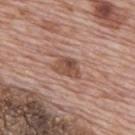Clinical impression: Recorded during total-body skin imaging; not selected for excision or biopsy. Image and clinical context: A 15 mm close-up extracted from a 3D total-body photography capture. Located on the upper back. The patient is a male aged around 70. Automated image analysis of the tile measured a color-variation rating of about 5/10 and a peripheral color-asymmetry measure near 2. It also reported a lesion-detection confidence of about 100/100. The tile uses white-light illumination. Approximately 3.5 mm at its widest.